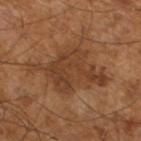workup: catalogued during a skin exam; not biopsied
body site: the leg
image: total-body-photography crop, ~15 mm field of view
lesion diameter: ~8 mm (longest diameter)
subject: male, in their mid- to late 60s
illumination: cross-polarized illumination